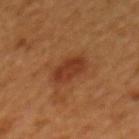Located on the back.
A male patient, in their mid- to late 40s.
The lesion-visualizer software estimated an area of roughly 7.5 mm², an outline eccentricity of about 0.75 (0 = round, 1 = elongated), and a symmetry-axis asymmetry near 0.15. The analysis additionally found a mean CIELAB color near L≈34 a*≈25 b*≈32, about 8 CIELAB-L* units darker than the surrounding skin, and a normalized border contrast of about 7.5. And it measured a within-lesion color-variation index near 2.5/10. It also reported a classifier nevus-likeness of about 75/100 and lesion-presence confidence of about 100/100.
A lesion tile, about 15 mm wide, cut from a 3D total-body photograph.
The recorded lesion diameter is about 3.5 mm.